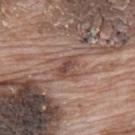Impression: Imaged during a routine full-body skin examination; the lesion was not biopsied and no histopathology is available. Context: The total-body-photography lesion software estimated an area of roughly 4.5 mm², a shape eccentricity near 0.75, and a symmetry-axis asymmetry near 0.25. The software also gave an average lesion color of about L≈47 a*≈19 b*≈24 (CIELAB) and a normalized border contrast of about 7. The software also gave a nevus-likeness score of about 0/100. A male patient, in their 70s. The lesion is located on the back. Imaged with white-light lighting. Longest diameter approximately 3 mm. Cropped from a total-body skin-imaging series; the visible field is about 15 mm.